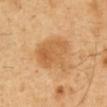The lesion was photographed on a routine skin check and not biopsied; there is no pathology result.
The tile uses cross-polarized illumination.
Measured at roughly 5.5 mm in maximum diameter.
A male patient, about 50 years old.
Automated tile analysis of the lesion measured a border-irregularity index near 3/10 and internal color variation of about 4 on a 0–10 scale. It also reported an automated nevus-likeness rating near 25 out of 100 and lesion-presence confidence of about 100/100.
A region of skin cropped from a whole-body photographic capture, roughly 15 mm wide.
Located on the abdomen.